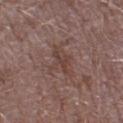Imaged during a routine full-body skin examination; the lesion was not biopsied and no histopathology is available. The total-body-photography lesion software estimated a footprint of about 3 mm² and an outline eccentricity of about 0.9 (0 = round, 1 = elongated). The software also gave an automated nevus-likeness rating near 0 out of 100. Measured at roughly 3 mm in maximum diameter. A lesion tile, about 15 mm wide, cut from a 3D total-body photograph. A male subject aged 43 to 47. The lesion is located on the leg.